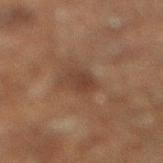From the right lower leg.
The recorded lesion diameter is about 3 mm.
A 15 mm crop from a total-body photograph taken for skin-cancer surveillance.
An algorithmic analysis of the crop reported an automated nevus-likeness rating near 0 out of 100 and a lesion-detection confidence of about 100/100.
A male patient, about 60 years old.
Imaged with cross-polarized lighting.